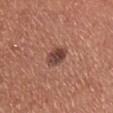Recorded during total-body skin imaging; not selected for excision or biopsy. A male subject, aged 68 to 72. From the right lower leg. A lesion tile, about 15 mm wide, cut from a 3D total-body photograph.